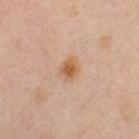  biopsy_status: not biopsied; imaged during a skin examination
  lighting: cross-polarized
  site: left thigh
  lesion_size:
    long_diameter_mm_approx: 2.5
  patient:
    sex: female
    age_approx: 40
  image:
    source: total-body photography crop
    field_of_view_mm: 15